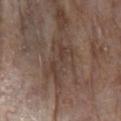No biopsy was performed on this lesion — it was imaged during a full skin examination and was not determined to be concerning. Automated tile analysis of the lesion measured an area of roughly 11 mm² and an outline eccentricity of about 0.8 (0 = round, 1 = elongated). And it measured an average lesion color of about L≈40 a*≈15 b*≈22 (CIELAB), a lesion–skin lightness drop of about 6, and a normalized lesion–skin contrast near 5.5. The analysis additionally found internal color variation of about 4 on a 0–10 scale. On the left forearm. A 15 mm close-up extracted from a 3D total-body photography capture. A female subject, aged approximately 85 or older. Longest diameter approximately 5.5 mm.